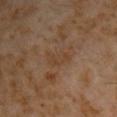Clinical impression: Captured during whole-body skin photography for melanoma surveillance; the lesion was not biopsied. Image and clinical context: The lesion's longest dimension is about 3.5 mm. The patient is a male roughly 60 years of age. From the right upper arm. A 15 mm close-up extracted from a 3D total-body photography capture.